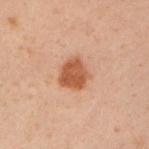Captured during whole-body skin photography for melanoma surveillance; the lesion was not biopsied.
An algorithmic analysis of the crop reported a lesion area of about 8 mm², an outline eccentricity of about 0.5 (0 = round, 1 = elongated), and a shape-asymmetry score of about 0.2 (0 = symmetric). The analysis additionally found roughly 11 lightness units darker than nearby skin and a normalized border contrast of about 9.5. The analysis additionally found border irregularity of about 2 on a 0–10 scale, internal color variation of about 2.5 on a 0–10 scale, and radial color variation of about 1.
The subject is a male aged 48 to 52.
About 3.5 mm across.
A 15 mm close-up extracted from a 3D total-body photography capture.
Imaged with cross-polarized lighting.
The lesion is on the left upper arm.